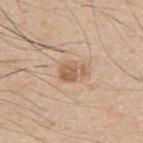Clinical impression: No biopsy was performed on this lesion — it was imaged during a full skin examination and was not determined to be concerning. Context: About 3 mm across. On the right upper arm. A region of skin cropped from a whole-body photographic capture, roughly 15 mm wide. This is a white-light tile. The subject is a male about 30 years old. The lesion-visualizer software estimated a border-irregularity index near 2.5/10, a within-lesion color-variation index near 2.5/10, and radial color variation of about 1.